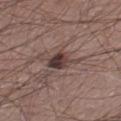Imaged during a routine full-body skin examination; the lesion was not biopsied and no histopathology is available. This is a white-light tile. A male subject approximately 55 years of age. Cropped from a total-body skin-imaging series; the visible field is about 15 mm. On the left thigh. Measured at roughly 3.5 mm in maximum diameter.